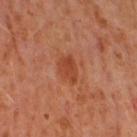Q: Was a biopsy performed?
A: imaged on a skin check; not biopsied